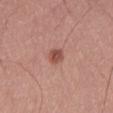Clinical impression: Imaged during a routine full-body skin examination; the lesion was not biopsied and no histopathology is available. Background: A 15 mm crop from a total-body photograph taken for skin-cancer surveillance. This is a white-light tile. Automated image analysis of the tile measured an area of roughly 3.5 mm², an eccentricity of roughly 0.8, and a shape-asymmetry score of about 0.3 (0 = symmetric). The software also gave a lesion-detection confidence of about 100/100. A male patient, about 70 years old. The lesion is located on the left thigh.